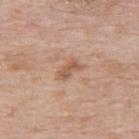The lesion was tiled from a total-body skin photograph and was not biopsied. The patient is a male aged around 75. From the upper back. The tile uses white-light illumination. A 15 mm crop from a total-body photograph taken for skin-cancer surveillance. About 3.5 mm across. Automated image analysis of the tile measured an average lesion color of about L≈57 a*≈20 b*≈31 (CIELAB) and a lesion-to-skin contrast of about 6.5 (normalized; higher = more distinct). And it measured a border-irregularity index near 3/10, internal color variation of about 3 on a 0–10 scale, and radial color variation of about 1.